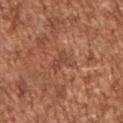Assessment:
Part of a total-body skin-imaging series; this lesion was reviewed on a skin check and was not flagged for biopsy.
Acquisition and patient details:
This image is a 15 mm lesion crop taken from a total-body photograph. On the right upper arm. The recorded lesion diameter is about 2.5 mm. The subject is a male about 45 years old. Captured under white-light illumination.